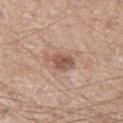| key | value |
|---|---|
| notes | catalogued during a skin exam; not biopsied |
| size | ~3.5 mm (longest diameter) |
| patient | male, roughly 65 years of age |
| acquisition | ~15 mm crop, total-body skin-cancer survey |
| site | the left thigh |
| automated metrics | a footprint of about 6.5 mm², an outline eccentricity of about 0.75 (0 = round, 1 = elongated), and a symmetry-axis asymmetry near 0.25; a lesion color around L≈54 a*≈20 b*≈26 in CIELAB and a lesion–skin lightness drop of about 11; border irregularity of about 3 on a 0–10 scale, internal color variation of about 3.5 on a 0–10 scale, and a peripheral color-asymmetry measure near 1 |
| tile lighting | white-light |